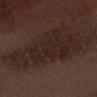follow-up=catalogued during a skin exam; not biopsied
patient=male, roughly 70 years of age
lesion diameter=~9 mm (longest diameter)
location=the right forearm
illumination=white-light
image=~15 mm crop, total-body skin-cancer survey